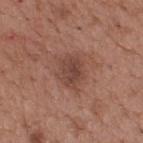No biopsy was performed on this lesion — it was imaged during a full skin examination and was not determined to be concerning. The lesion is located on the upper back. A 15 mm crop from a total-body photograph taken for skin-cancer surveillance. Longest diameter approximately 3.5 mm. A male subject approximately 65 years of age.